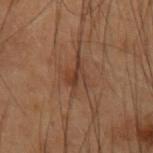Captured during whole-body skin photography for melanoma surveillance; the lesion was not biopsied. A male subject roughly 55 years of age. Captured under cross-polarized illumination. Measured at roughly 3.5 mm in maximum diameter. Automated image analysis of the tile measured a lesion color around L≈31 a*≈16 b*≈24 in CIELAB and a normalized border contrast of about 6. A roughly 15 mm field-of-view crop from a total-body skin photograph. The lesion is located on the right forearm.